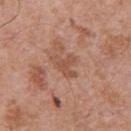Notes:
- follow-up — no biopsy performed (imaged during a skin exam)
- imaging modality — ~15 mm tile from a whole-body skin photo
- subject — male, approximately 70 years of age
- location — the chest